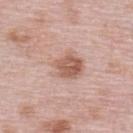workup = imaged on a skin check; not biopsied
subject = female, approximately 65 years of age
anatomic site = the upper back
automated metrics = a classifier nevus-likeness of about 45/100 and a detector confidence of about 100 out of 100 that the crop contains a lesion
lesion size = ≈5.5 mm
acquisition = total-body-photography crop, ~15 mm field of view
illumination = white-light